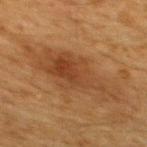Clinical impression: The lesion was photographed on a routine skin check and not biopsied; there is no pathology result. Acquisition and patient details: Automated tile analysis of the lesion measured a footprint of about 21 mm², an eccentricity of roughly 0.85, and two-axis asymmetry of about 0.4. The analysis additionally found a mean CIELAB color near L≈36 a*≈19 b*≈31 and a normalized border contrast of about 6.5. The analysis additionally found a within-lesion color-variation index near 4.5/10 and a peripheral color-asymmetry measure near 1.5. From the upper back. A male subject roughly 60 years of age. A 15 mm close-up tile from a total-body photography series done for melanoma screening. About 8 mm across.